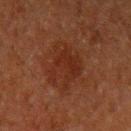{"biopsy_status": "not biopsied; imaged during a skin examination", "lighting": "cross-polarized", "patient": {"sex": "male", "age_approx": 60}, "image": {"source": "total-body photography crop", "field_of_view_mm": 15}, "lesion_size": {"long_diameter_mm_approx": 5.5}, "automated_metrics": {"border_irregularity_0_10": 5.0, "color_variation_0_10": 2.5, "peripheral_color_asymmetry": 1.0, "nevus_likeness_0_100": 15}, "site": "arm"}